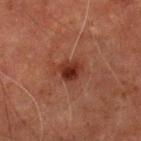Imaged during a routine full-body skin examination; the lesion was not biopsied and no histopathology is available.
A male subject, aged 68 to 72.
The lesion is on the left lower leg.
A 15 mm close-up tile from a total-body photography series done for melanoma screening.